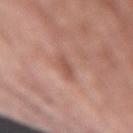No biopsy was performed on this lesion — it was imaged during a full skin examination and was not determined to be concerning.
Measured at roughly 2.5 mm in maximum diameter.
The lesion is on the left upper arm.
An algorithmic analysis of the crop reported a lesion color around L≈53 a*≈23 b*≈27 in CIELAB and about 9 CIELAB-L* units darker than the surrounding skin. The software also gave an automated nevus-likeness rating near 0 out of 100.
The patient is a female aged approximately 70.
Imaged with white-light lighting.
Cropped from a total-body skin-imaging series; the visible field is about 15 mm.